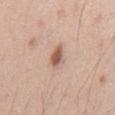– biopsy status — imaged on a skin check; not biopsied
– subject — male, about 50 years old
– lesion size — ~3 mm (longest diameter)
– illumination — white-light
– image source — ~15 mm crop, total-body skin-cancer survey
– location — the front of the torso